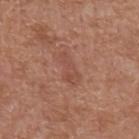| feature | finding |
|---|---|
| workup | catalogued during a skin exam; not biopsied |
| location | the abdomen |
| subject | male, in their mid-60s |
| tile lighting | white-light |
| image source | ~15 mm crop, total-body skin-cancer survey |
| diameter | ~4 mm (longest diameter) |